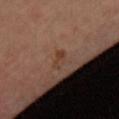workup: catalogued during a skin exam; not biopsied
image source: total-body-photography crop, ~15 mm field of view
tile lighting: cross-polarized
patient: female, approximately 45 years of age
location: the chest
diameter: ~2.5 mm (longest diameter)